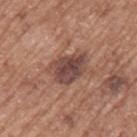Q: Is there a histopathology result?
A: imaged on a skin check; not biopsied
Q: What are the patient's age and sex?
A: male, aged 73–77
Q: Where on the body is the lesion?
A: the left upper arm
Q: How large is the lesion?
A: ≈4.5 mm
Q: What lighting was used for the tile?
A: white-light illumination
Q: Automated lesion metrics?
A: roughly 12 lightness units darker than nearby skin and a lesion-to-skin contrast of about 9.5 (normalized; higher = more distinct); a color-variation rating of about 5/10 and radial color variation of about 1.5; lesion-presence confidence of about 100/100
Q: How was this image acquired?
A: ~15 mm crop, total-body skin-cancer survey